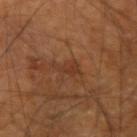biopsy status: imaged on a skin check; not biopsied
illumination: cross-polarized illumination
TBP lesion metrics: a lesion area of about 4.5 mm², an eccentricity of roughly 0.85, and a shape-asymmetry score of about 0.45 (0 = symmetric); an average lesion color of about L≈32 a*≈20 b*≈29 (CIELAB); a within-lesion color-variation index near 1.5/10 and peripheral color asymmetry of about 0.5
image source: total-body-photography crop, ~15 mm field of view
subject: male, aged 58 to 62
location: the right forearm